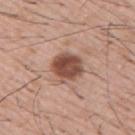The lesion was photographed on a routine skin check and not biopsied; there is no pathology result. The total-body-photography lesion software estimated a footprint of about 11 mm² and a shape eccentricity near 0.4. The analysis additionally found an average lesion color of about L≈49 a*≈21 b*≈27 (CIELAB), about 15 CIELAB-L* units darker than the surrounding skin, and a normalized border contrast of about 10.5. It also reported a classifier nevus-likeness of about 75/100. Cropped from a total-body skin-imaging series; the visible field is about 15 mm. A male subject aged around 55.